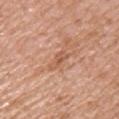The lesion was tiled from a total-body skin photograph and was not biopsied. From the chest. Measured at roughly 3 mm in maximum diameter. Imaged with white-light lighting. A male subject, aged 58 to 62. A region of skin cropped from a whole-body photographic capture, roughly 15 mm wide.